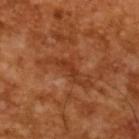workup=no biopsy performed (imaged during a skin exam)
image source=~15 mm crop, total-body skin-cancer survey
patient=male, in their mid-60s
TBP lesion metrics=a lesion–skin lightness drop of about 7; an automated nevus-likeness rating near 0 out of 100 and a detector confidence of about 65 out of 100 that the crop contains a lesion